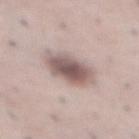<case>
  <biopsy_status>not biopsied; imaged during a skin examination</biopsy_status>
  <site>abdomen</site>
  <automated_metrics>
    <vs_skin_darker_L>15.0</vs_skin_darker_L>
    <vs_skin_contrast_norm>9.5</vs_skin_contrast_norm>
    <nevus_likeness_0_100>45</nevus_likeness_0_100>
    <lesion_detection_confidence_0_100>100</lesion_detection_confidence_0_100>
  </automated_metrics>
  <patient>
    <sex>male</sex>
    <age_approx>50</age_approx>
  </patient>
  <lesion_size>
    <long_diameter_mm_approx>4.5</long_diameter_mm_approx>
  </lesion_size>
  <image>
    <source>total-body photography crop</source>
    <field_of_view_mm>15</field_of_view_mm>
  </image>
</case>